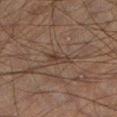Recorded during total-body skin imaging; not selected for excision or biopsy.
This is a cross-polarized tile.
A male patient, aged around 50.
About 3 mm across.
The total-body-photography lesion software estimated a lesion area of about 3 mm², a shape eccentricity near 0.95, and two-axis asymmetry of about 0.4. It also reported a lesion color around L≈28 a*≈12 b*≈19 in CIELAB and a normalized lesion–skin contrast near 6.
This image is a 15 mm lesion crop taken from a total-body photograph.
The lesion is located on the left lower leg.